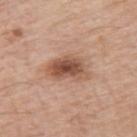{
  "biopsy_status": "not biopsied; imaged during a skin examination",
  "patient": {
    "sex": "male",
    "age_approx": 75
  },
  "image": {
    "source": "total-body photography crop",
    "field_of_view_mm": 15
  },
  "site": "arm"
}